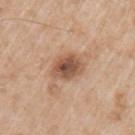Q: Was this lesion biopsied?
A: total-body-photography surveillance lesion; no biopsy
Q: Lesion location?
A: the left upper arm
Q: What did automated image analysis measure?
A: a footprint of about 9 mm² and a shape-asymmetry score of about 0.15 (0 = symmetric); a border-irregularity index near 1.5/10, internal color variation of about 6 on a 0–10 scale, and peripheral color asymmetry of about 1.5
Q: What kind of image is this?
A: ~15 mm crop, total-body skin-cancer survey
Q: Illumination type?
A: white-light
Q: What are the patient's age and sex?
A: male, about 65 years old